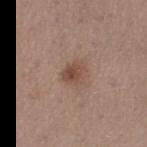Recorded during total-body skin imaging; not selected for excision or biopsy.
A female subject in their 30s.
The lesion is located on the left thigh.
Imaged with white-light lighting.
A 15 mm crop from a total-body photograph taken for skin-cancer surveillance.